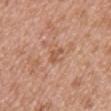The lesion was tiled from a total-body skin photograph and was not biopsied. Imaged with white-light lighting. The lesion is on the chest. Approximately 2.5 mm at its widest. A female patient aged 38–42. A 15 mm crop from a total-body photograph taken for skin-cancer surveillance. Automated tile analysis of the lesion measured an average lesion color of about L≈55 a*≈23 b*≈32 (CIELAB), a lesion–skin lightness drop of about 8, and a normalized border contrast of about 6. It also reported a border-irregularity rating of about 5/10 and a color-variation rating of about 0/10.